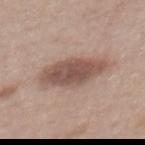Assessment:
The lesion was photographed on a routine skin check and not biopsied; there is no pathology result.
Image and clinical context:
A female subject about 55 years old. Cropped from a total-body skin-imaging series; the visible field is about 15 mm. Located on the back.